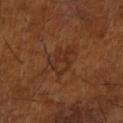Impression: Part of a total-body skin-imaging series; this lesion was reviewed on a skin check and was not flagged for biopsy. Background: A region of skin cropped from a whole-body photographic capture, roughly 15 mm wide. The patient is a male aged 63 to 67. Automated tile analysis of the lesion measured a lesion area of about 8 mm², a shape eccentricity near 0.8, and a symmetry-axis asymmetry near 0.4. And it measured border irregularity of about 5.5 on a 0–10 scale, internal color variation of about 3.5 on a 0–10 scale, and radial color variation of about 1. It also reported an automated nevus-likeness rating near 0 out of 100 and lesion-presence confidence of about 100/100. This is a cross-polarized tile. From the right upper arm.